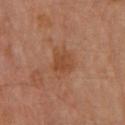Case summary:
– acquisition — ~15 mm tile from a whole-body skin photo
– location — the left forearm
– lighting — cross-polarized
– lesion size — ≈2.5 mm
– subject — female, aged 63–67